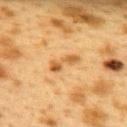notes — no biopsy performed (imaged during a skin exam) | lighting — cross-polarized | patient — female, approximately 40 years of age | acquisition — 15 mm crop, total-body photography | size — about 4 mm | body site — the upper back | image-analysis metrics — an eccentricity of roughly 0.95 and two-axis asymmetry of about 0.4; an average lesion color of about L≈49 a*≈19 b*≈39 (CIELAB), a lesion–skin lightness drop of about 10, and a normalized border contrast of about 8; border irregularity of about 5.5 on a 0–10 scale, a color-variation rating of about 3.5/10, and a peripheral color-asymmetry measure near 1.